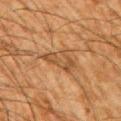Imaged during a routine full-body skin examination; the lesion was not biopsied and no histopathology is available.
The total-body-photography lesion software estimated a nevus-likeness score of about 0/100 and lesion-presence confidence of about 65/100.
A 15 mm close-up tile from a total-body photography series done for melanoma screening.
On the right upper arm.
Imaged with cross-polarized lighting.
The subject is a male aged around 65.